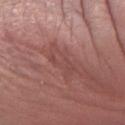Impression:
Imaged during a routine full-body skin examination; the lesion was not biopsied and no histopathology is available.
Context:
A male subject aged 73–77. Captured under white-light illumination. The total-body-photography lesion software estimated an area of roughly 7.5 mm² and a shape-asymmetry score of about 0.25 (0 = symmetric). The software also gave roughly 6 lightness units darker than nearby skin. And it measured a border-irregularity index near 3.5/10 and radial color variation of about 1. On the left forearm. Longest diameter approximately 4.5 mm. A close-up tile cropped from a whole-body skin photograph, about 15 mm across.